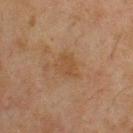The lesion was photographed on a routine skin check and not biopsied; there is no pathology result.
Measured at roughly 3.5 mm in maximum diameter.
This image is a 15 mm lesion crop taken from a total-body photograph.
This is a cross-polarized tile.
The lesion is located on the upper back.
A male subject in their mid- to late 40s.